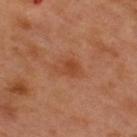| key | value |
|---|---|
| notes | total-body-photography surveillance lesion; no biopsy |
| image source | ~15 mm tile from a whole-body skin photo |
| anatomic site | the upper back |
| lighting | cross-polarized |
| patient | male, roughly 50 years of age |
| automated lesion analysis | an area of roughly 5 mm², an eccentricity of roughly 0.75, and a symmetry-axis asymmetry near 0.4; a mean CIELAB color near L≈45 a*≈26 b*≈35, about 7 CIELAB-L* units darker than the surrounding skin, and a normalized lesion–skin contrast near 6; a border-irregularity rating of about 4/10, a within-lesion color-variation index near 2/10, and a peripheral color-asymmetry measure near 0.5; an automated nevus-likeness rating near 5 out of 100 and a lesion-detection confidence of about 100/100 |